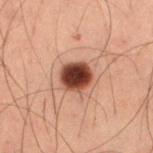Findings:
- follow-up · catalogued during a skin exam; not biopsied
- tile lighting · cross-polarized illumination
- patient · male, roughly 60 years of age
- lesion diameter · about 3.5 mm
- imaging modality · 15 mm crop, total-body photography
- body site · the right thigh